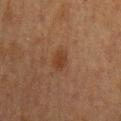Part of a total-body skin-imaging series; this lesion was reviewed on a skin check and was not flagged for biopsy. A male patient, in their mid- to late 70s. The lesion is located on the back. A roughly 15 mm field-of-view crop from a total-body skin photograph.